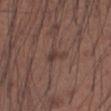| field | value |
|---|---|
| notes | catalogued during a skin exam; not biopsied |
| patient | male, in their mid-30s |
| tile lighting | white-light |
| TBP lesion metrics | a lesion area of about 3 mm²; an average lesion color of about L≈38 a*≈17 b*≈20 (CIELAB) and roughly 7 lightness units darker than nearby skin |
| imaging modality | ~15 mm tile from a whole-body skin photo |
| site | the left forearm |